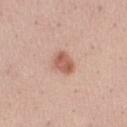Q: Is there a histopathology result?
A: imaged on a skin check; not biopsied
Q: What is the imaging modality?
A: total-body-photography crop, ~15 mm field of view
Q: What are the patient's age and sex?
A: female, approximately 25 years of age
Q: What did automated image analysis measure?
A: a border-irregularity index near 1.5/10 and a peripheral color-asymmetry measure near 1
Q: What is the anatomic site?
A: the leg
Q: What is the lesion's diameter?
A: ~3 mm (longest diameter)